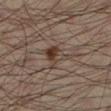Q: Was this lesion biopsied?
A: no biopsy performed (imaged during a skin exam)
Q: Lesion size?
A: ≈4 mm
Q: What kind of image is this?
A: total-body-photography crop, ~15 mm field of view
Q: Lesion location?
A: the left lower leg
Q: Automated lesion metrics?
A: a lesion–skin lightness drop of about 8 and a normalized border contrast of about 8; a border-irregularity index near 5.5/10, internal color variation of about 7 on a 0–10 scale, and peripheral color asymmetry of about 2.5
Q: Patient demographics?
A: male, aged 33 to 37